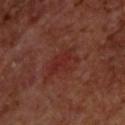image source: ~15 mm tile from a whole-body skin photo
anatomic site: the upper back
TBP lesion metrics: a lesion–skin lightness drop of about 5 and a normalized lesion–skin contrast near 5.5; a border-irregularity rating of about 5.5/10, a within-lesion color-variation index near 2.5/10, and a peripheral color-asymmetry measure near 1
illumination: cross-polarized
patient: male, aged 68 to 72
lesion diameter: ~4.5 mm (longest diameter)